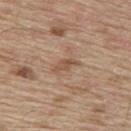workup — no biopsy performed (imaged during a skin exam)
image — 15 mm crop, total-body photography
automated metrics — a lesion area of about 3 mm²; a lesion color around L≈52 a*≈18 b*≈31 in CIELAB, roughly 9 lightness units darker than nearby skin, and a normalized lesion–skin contrast near 6.5; an automated nevus-likeness rating near 0 out of 100
anatomic site — the mid back
subject — male, roughly 70 years of age
diameter — ≈3 mm
lighting — white-light illumination